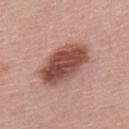notes: total-body-photography surveillance lesion; no biopsy
imaging modality: ~15 mm tile from a whole-body skin photo
patient: male, in their 60s
anatomic site: the mid back